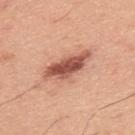Imaged during a routine full-body skin examination; the lesion was not biopsied and no histopathology is available.
This is a white-light tile.
A male patient approximately 45 years of age.
A lesion tile, about 15 mm wide, cut from a 3D total-body photograph.
From the upper back.
The lesion-visualizer software estimated an area of roughly 13 mm². The software also gave a border-irregularity index near 3.5/10, internal color variation of about 6 on a 0–10 scale, and radial color variation of about 2. The software also gave a classifier nevus-likeness of about 90/100 and lesion-presence confidence of about 100/100.